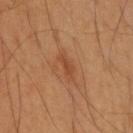Impression: Captured during whole-body skin photography for melanoma surveillance; the lesion was not biopsied. Clinical summary: This is a cross-polarized tile. This image is a 15 mm lesion crop taken from a total-body photograph. The recorded lesion diameter is about 3.5 mm. A male subject, about 55 years old. The lesion-visualizer software estimated an eccentricity of roughly 0.95 and two-axis asymmetry of about 0.35. It also reported an average lesion color of about L≈45 a*≈23 b*≈35 (CIELAB), roughly 7 lightness units darker than nearby skin, and a normalized lesion–skin contrast near 5.5. The analysis additionally found radial color variation of about 0. The lesion is located on the back.